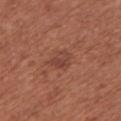Clinical impression:
Imaged during a routine full-body skin examination; the lesion was not biopsied and no histopathology is available.
Clinical summary:
A female patient roughly 65 years of age. A 15 mm close-up tile from a total-body photography series done for melanoma screening. Automated tile analysis of the lesion measured a lesion area of about 5 mm² and a shape-asymmetry score of about 0.3 (0 = symmetric). The software also gave an average lesion color of about L≈43 a*≈25 b*≈27 (CIELAB), roughly 7 lightness units darker than nearby skin, and a normalized lesion–skin contrast near 5.5. The analysis additionally found a classifier nevus-likeness of about 20/100 and a lesion-detection confidence of about 100/100. The recorded lesion diameter is about 3 mm. The lesion is located on the back.